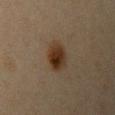| key | value |
|---|---|
| workup | no biopsy performed (imaged during a skin exam) |
| illumination | cross-polarized illumination |
| lesion size | ~3.5 mm (longest diameter) |
| image source | ~15 mm crop, total-body skin-cancer survey |
| patient | female, aged approximately 45 |
| location | the arm |